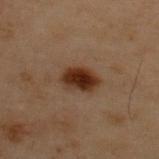Impression: Imaged during a routine full-body skin examination; the lesion was not biopsied and no histopathology is available. Acquisition and patient details: The patient is a male approximately 55 years of age. The tile uses cross-polarized illumination. A 15 mm crop from a total-body photograph taken for skin-cancer surveillance. Measured at roughly 4 mm in maximum diameter. The lesion is on the upper back.